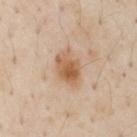Q: Was this lesion biopsied?
A: imaged on a skin check; not biopsied
Q: Lesion size?
A: ~4.5 mm (longest diameter)
Q: Where on the body is the lesion?
A: the chest
Q: What kind of image is this?
A: ~15 mm tile from a whole-body skin photo
Q: How was the tile lit?
A: cross-polarized illumination
Q: Who is the patient?
A: male, roughly 55 years of age